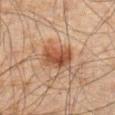biopsy_status: not biopsied; imaged during a skin examination
site: abdomen
image:
  source: total-body photography crop
  field_of_view_mm: 15
lighting: cross-polarized
lesion_size:
  long_diameter_mm_approx: 4.0
patient:
  sex: male
  age_approx: 65